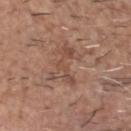Recorded during total-body skin imaging; not selected for excision or biopsy.
Longest diameter approximately 5 mm.
Cropped from a whole-body photographic skin survey; the tile spans about 15 mm.
Automated tile analysis of the lesion measured a footprint of about 8 mm², an outline eccentricity of about 0.85 (0 = round, 1 = elongated), and a shape-asymmetry score of about 0.6 (0 = symmetric). The software also gave border irregularity of about 9 on a 0–10 scale and a within-lesion color-variation index near 3/10. It also reported a lesion-detection confidence of about 90/100.
From the head or neck.
A male patient, aged 68 to 72.